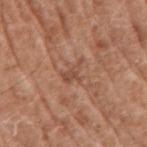Imaged during a routine full-body skin examination; the lesion was not biopsied and no histopathology is available.
A region of skin cropped from a whole-body photographic capture, roughly 15 mm wide.
This is a white-light tile.
About 3 mm across.
The subject is a male in their mid- to late 70s.
The lesion is on the right upper arm.